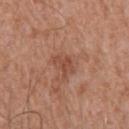workup: imaged on a skin check; not biopsied | lighting: white-light illumination | location: the upper back | imaging modality: ~15 mm tile from a whole-body skin photo | size: ~3 mm (longest diameter) | patient: male, in their 60s | automated metrics: a shape eccentricity near 0.75; a border-irregularity rating of about 5/10, a color-variation rating of about 1/10, and a peripheral color-asymmetry measure near 0.5; a classifier nevus-likeness of about 0/100 and a detector confidence of about 100 out of 100 that the crop contains a lesion.